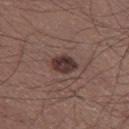Case summary:
* notes — imaged on a skin check; not biopsied
* subject — male, aged 53–57
* acquisition — ~15 mm tile from a whole-body skin photo
* site — the left thigh